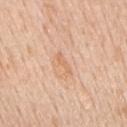notes = total-body-photography surveillance lesion; no biopsy.